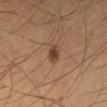biopsy status = catalogued during a skin exam; not biopsied
imaging modality = 15 mm crop, total-body photography
TBP lesion metrics = a classifier nevus-likeness of about 95/100
tile lighting = cross-polarized
diameter = ≈2 mm
body site = the left lower leg
patient = male, aged 28 to 32